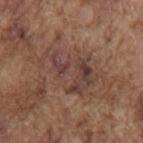<lesion>
  <biopsy_status>not biopsied; imaged during a skin examination</biopsy_status>
  <image>
    <source>total-body photography crop</source>
    <field_of_view_mm>15</field_of_view_mm>
  </image>
  <automated_metrics>
    <cielab_L>41</cielab_L>
    <cielab_a>18</cielab_a>
    <cielab_b>23</cielab_b>
    <nevus_likeness_0_100>0</nevus_likeness_0_100>
    <lesion_detection_confidence_0_100>65</lesion_detection_confidence_0_100>
  </automated_metrics>
  <patient>
    <sex>male</sex>
    <age_approx>75</age_approx>
  </patient>
  <lesion_size>
    <long_diameter_mm_approx>7.0</long_diameter_mm_approx>
  </lesion_size>
  <site>back</site>
</lesion>